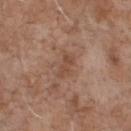Case summary:
* workup · imaged on a skin check; not biopsied
* acquisition · 15 mm crop, total-body photography
* site · the front of the torso
* TBP lesion metrics · about 7 CIELAB-L* units darker than the surrounding skin and a normalized lesion–skin contrast near 6; a border-irregularity rating of about 4/10, internal color variation of about 2 on a 0–10 scale, and peripheral color asymmetry of about 0.5
* lighting · white-light
* subject · male, aged 68–72
* lesion size · ≈3 mm